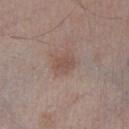workup: no biopsy performed (imaged during a skin exam); imaging modality: ~15 mm tile from a whole-body skin photo; body site: the leg; lesion diameter: about 2.5 mm; subject: male, aged 53–57; illumination: white-light illumination.